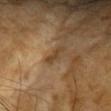follow-up = imaged on a skin check; not biopsied | acquisition = total-body-photography crop, ~15 mm field of view | lesion size = ~3.5 mm (longest diameter) | illumination = cross-polarized | location = the head or neck | patient = female, aged approximately 60.